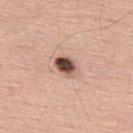notes: total-body-photography surveillance lesion; no biopsy | patient: male, aged approximately 65 | location: the upper back | TBP lesion metrics: about 20 CIELAB-L* units darker than the surrounding skin | image: 15 mm crop, total-body photography | lesion diameter: ~3 mm (longest diameter) | tile lighting: white-light illumination.